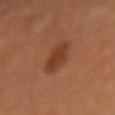<lesion>
  <biopsy_status>not biopsied; imaged during a skin examination</biopsy_status>
  <site>right forearm</site>
  <automated_metrics>
    <eccentricity>0.5</eccentricity>
    <shape_asymmetry>0.15</shape_asymmetry>
    <cielab_L>37</cielab_L>
    <cielab_a>24</cielab_a>
    <cielab_b>31</cielab_b>
    <vs_skin_darker_L>9.0</vs_skin_darker_L>
    <vs_skin_contrast_norm>7.5</vs_skin_contrast_norm>
    <border_irregularity_0_10>2.0</border_irregularity_0_10>
    <color_variation_0_10>2.0</color_variation_0_10>
    <peripheral_color_asymmetry>1.0</peripheral_color_asymmetry>
    <nevus_likeness_0_100>80</nevus_likeness_0_100>
    <lesion_detection_confidence_0_100>100</lesion_detection_confidence_0_100>
  </automated_metrics>
  <patient>
    <sex>female</sex>
    <age_approx>35</age_approx>
  </patient>
  <lighting>cross-polarized</lighting>
  <lesion_size>
    <long_diameter_mm_approx>3.0</long_diameter_mm_approx>
  </lesion_size>
  <image>
    <source>total-body photography crop</source>
    <field_of_view_mm>15</field_of_view_mm>
  </image>
</lesion>